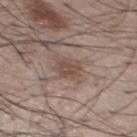Findings:
- workup · total-body-photography surveillance lesion; no biopsy
- imaging modality · ~15 mm crop, total-body skin-cancer survey
- anatomic site · the chest
- patient · male, aged approximately 55
- lighting · white-light illumination